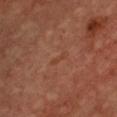| feature | finding |
|---|---|
| location | the chest |
| diameter | ≈2.5 mm |
| acquisition | total-body-photography crop, ~15 mm field of view |
| tile lighting | cross-polarized |
| patient | female, aged 43–47 |